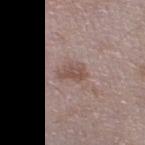{"biopsy_status": "not biopsied; imaged during a skin examination", "site": "left thigh", "patient": {"sex": "male", "age_approx": 65}, "image": {"source": "total-body photography crop", "field_of_view_mm": 15}}